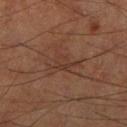Case summary:
* follow-up · total-body-photography surveillance lesion; no biopsy
* lighting · cross-polarized
* site · the leg
* acquisition · ~15 mm tile from a whole-body skin photo
* subject · male, aged 63 to 67